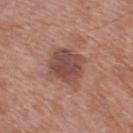Findings:
* notes: catalogued during a skin exam; not biopsied
* diameter: about 4.5 mm
* body site: the left lower leg
* tile lighting: white-light illumination
* acquisition: ~15 mm crop, total-body skin-cancer survey
* subject: male, aged 53–57